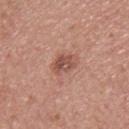- follow-up: no biopsy performed (imaged during a skin exam)